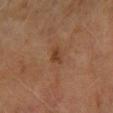Clinical impression: Captured during whole-body skin photography for melanoma surveillance; the lesion was not biopsied. Acquisition and patient details: Measured at roughly 2.5 mm in maximum diameter. Captured under cross-polarized illumination. A region of skin cropped from a whole-body photographic capture, roughly 15 mm wide. Automated image analysis of the tile measured a footprint of about 3 mm², an eccentricity of roughly 0.85, and a shape-asymmetry score of about 0.35 (0 = symmetric). And it measured an average lesion color of about L≈36 a*≈20 b*≈30 (CIELAB) and about 7 CIELAB-L* units darker than the surrounding skin. The subject is a female aged around 60. From the left forearm.